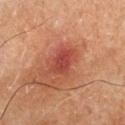biopsy status: imaged on a skin check; not biopsied | location: the right lower leg | size: ≈3 mm | image: ~15 mm crop, total-body skin-cancer survey | automated lesion analysis: a mean CIELAB color near L≈36 a*≈28 b*≈25, a lesion–skin lightness drop of about 8, and a lesion-to-skin contrast of about 7 (normalized; higher = more distinct); a border-irregularity index near 2.5/10, internal color variation of about 3.5 on a 0–10 scale, and radial color variation of about 1.5; a nevus-likeness score of about 60/100 and a detector confidence of about 100 out of 100 that the crop contains a lesion | tile lighting: cross-polarized illumination | patient: male, aged 63–67.